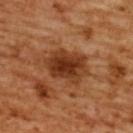A close-up tile cropped from a whole-body skin photograph, about 15 mm across. Located on the upper back. A female subject approximately 55 years of age.